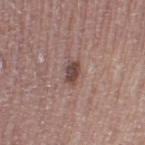Acquisition and patient details: The subject is a male in their mid-60s. Imaged with white-light lighting. Cropped from a total-body skin-imaging series; the visible field is about 15 mm. The lesion is located on the leg. The recorded lesion diameter is about 2.5 mm.